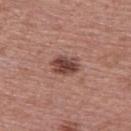Findings:
* follow-up — total-body-photography surveillance lesion; no biopsy
* subject — male, aged 53–57
* image source — total-body-photography crop, ~15 mm field of view
* body site — the upper back
* lesion diameter — ~3.5 mm (longest diameter)
* illumination — white-light illumination